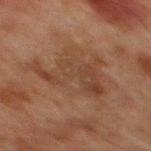Impression:
Captured during whole-body skin photography for melanoma surveillance; the lesion was not biopsied.
Clinical summary:
The tile uses cross-polarized illumination. From the chest. A 15 mm crop from a total-body photograph taken for skin-cancer surveillance. A male subject, aged 63 to 67. Approximately 7.5 mm at its widest.